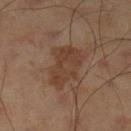This lesion was catalogued during total-body skin photography and was not selected for biopsy.